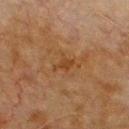Q: Is there a histopathology result?
A: no biopsy performed (imaged during a skin exam)
Q: How was the tile lit?
A: cross-polarized illumination
Q: What kind of image is this?
A: ~15 mm crop, total-body skin-cancer survey
Q: Where on the body is the lesion?
A: the chest
Q: Lesion size?
A: about 3 mm
Q: What are the patient's age and sex?
A: male, about 80 years old
Q: Automated lesion metrics?
A: a lesion area of about 3 mm², a shape eccentricity near 0.85, and two-axis asymmetry of about 0.35; an average lesion color of about L≈33 a*≈18 b*≈30 (CIELAB), about 5 CIELAB-L* units darker than the surrounding skin, and a normalized border contrast of about 6; border irregularity of about 4 on a 0–10 scale, a color-variation rating of about 1/10, and peripheral color asymmetry of about 0; a detector confidence of about 100 out of 100 that the crop contains a lesion